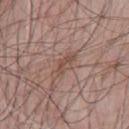workup: imaged on a skin check; not biopsied
location: the chest
illumination: white-light illumination
acquisition: total-body-photography crop, ~15 mm field of view
subject: male, aged 63 to 67
diameter: ~4 mm (longest diameter)